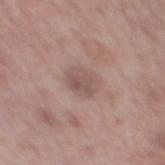– follow-up: imaged on a skin check; not biopsied
– patient: male, aged approximately 65
– imaging modality: 15 mm crop, total-body photography
– lighting: white-light
– anatomic site: the mid back
– image-analysis metrics: a lesion area of about 6.5 mm² and a symmetry-axis asymmetry near 0.15; a mean CIELAB color near L≈53 a*≈17 b*≈21, roughly 8 lightness units darker than nearby skin, and a normalized lesion–skin contrast near 6; border irregularity of about 1.5 on a 0–10 scale, a within-lesion color-variation index near 3/10, and a peripheral color-asymmetry measure near 1; lesion-presence confidence of about 100/100